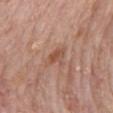Imaged during a routine full-body skin examination; the lesion was not biopsied and no histopathology is available. A close-up tile cropped from a whole-body skin photograph, about 15 mm across. This is a white-light tile. The lesion is located on the back. Longest diameter approximately 2.5 mm. The subject is a male aged approximately 75.